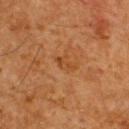Clinical impression:
Captured during whole-body skin photography for melanoma surveillance; the lesion was not biopsied.
Image and clinical context:
About 3 mm across. A region of skin cropped from a whole-body photographic capture, roughly 15 mm wide. The lesion is on the upper back. A male subject about 60 years old.